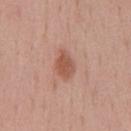<tbp_lesion>
  <biopsy_status>not biopsied; imaged during a skin examination</biopsy_status>
  <lighting>white-light</lighting>
  <lesion_size>
    <long_diameter_mm_approx>3.5</long_diameter_mm_approx>
  </lesion_size>
  <image>
    <source>total-body photography crop</source>
    <field_of_view_mm>15</field_of_view_mm>
  </image>
  <patient>
    <sex>male</sex>
    <age_approx>55</age_approx>
  </patient>
  <site>chest</site>
</tbp_lesion>